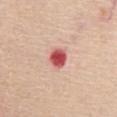Image and clinical context:
A female subject in their 70s. The tile uses white-light illumination. A 15 mm close-up extracted from a 3D total-body photography capture. The lesion is located on the chest.